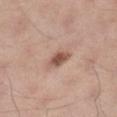| field | value |
|---|---|
| follow-up | total-body-photography surveillance lesion; no biopsy |
| image source | ~15 mm tile from a whole-body skin photo |
| lesion diameter | about 2.5 mm |
| tile lighting | white-light |
| subject | male, aged around 35 |
| location | the leg |